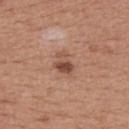| feature | finding |
|---|---|
| notes | no biopsy performed (imaged during a skin exam) |
| patient | female, aged approximately 35 |
| image-analysis metrics | a footprint of about 5 mm² and two-axis asymmetry of about 0.35; an average lesion color of about L≈50 a*≈22 b*≈29 (CIELAB), a lesion–skin lightness drop of about 11, and a lesion-to-skin contrast of about 7.5 (normalized; higher = more distinct); border irregularity of about 3.5 on a 0–10 scale and internal color variation of about 5.5 on a 0–10 scale; a nevus-likeness score of about 55/100 and a detector confidence of about 100 out of 100 that the crop contains a lesion |
| image source | ~15 mm crop, total-body skin-cancer survey |
| body site | the upper back |
| lesion size | ~3 mm (longest diameter) |
| lighting | white-light |